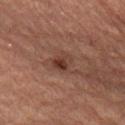workup: catalogued during a skin exam; not biopsied | diameter: about 2.5 mm | site: the leg | patient: female, in their 70s | lighting: cross-polarized illumination | imaging modality: total-body-photography crop, ~15 mm field of view.